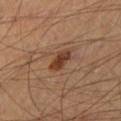{"biopsy_status": "not biopsied; imaged during a skin examination", "patient": {"sex": "male", "age_approx": 65}, "lesion_size": {"long_diameter_mm_approx": 3.5}, "image": {"source": "total-body photography crop", "field_of_view_mm": 15}, "lighting": "cross-polarized", "site": "leg", "automated_metrics": {"cielab_L": 40, "cielab_a": 21, "cielab_b": 30, "vs_skin_darker_L": 11.0, "vs_skin_contrast_norm": 9.0}}